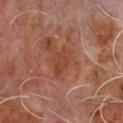Case summary:
• notes: total-body-photography surveillance lesion; no biopsy
• illumination: cross-polarized
• patient: male, in their mid-60s
• size: ≈3.5 mm
• anatomic site: the chest
• TBP lesion metrics: an area of roughly 5.5 mm² and a shape-asymmetry score of about 0.3 (0 = symmetric); a mean CIELAB color near L≈41 a*≈25 b*≈31 and a normalized border contrast of about 6; a detector confidence of about 100 out of 100 that the crop contains a lesion
• acquisition: ~15 mm crop, total-body skin-cancer survey